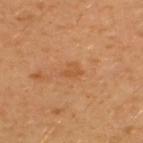{
  "biopsy_status": "not biopsied; imaged during a skin examination",
  "image": {
    "source": "total-body photography crop",
    "field_of_view_mm": 15
  },
  "site": "upper back",
  "lighting": "cross-polarized",
  "lesion_size": {
    "long_diameter_mm_approx": 2.5
  },
  "patient": {
    "sex": "male",
    "age_approx": 30
  }
}